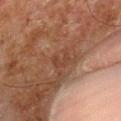Impression: The lesion was tiled from a total-body skin photograph and was not biopsied. Acquisition and patient details: The total-body-photography lesion software estimated a mean CIELAB color near L≈32 a*≈18 b*≈24. Captured under cross-polarized illumination. The lesion is located on the left thigh. The lesion's longest dimension is about 2.5 mm. A region of skin cropped from a whole-body photographic capture, roughly 15 mm wide. The subject is a male in their 80s.